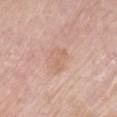biopsy status: no biopsy performed (imaged during a skin exam)
patient: male, aged 73 to 77
imaging modality: ~15 mm tile from a whole-body skin photo
diameter: about 3 mm
automated lesion analysis: a footprint of about 5 mm² and a symmetry-axis asymmetry near 0.35; an average lesion color of about L≈64 a*≈20 b*≈30 (CIELAB) and about 6 CIELAB-L* units darker than the surrounding skin; a within-lesion color-variation index near 2/10 and peripheral color asymmetry of about 1; a classifier nevus-likeness of about 0/100 and a detector confidence of about 100 out of 100 that the crop contains a lesion
anatomic site: the chest